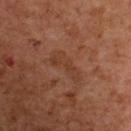workup: total-body-photography surveillance lesion; no biopsy | body site: the upper back | lesion size: ≈4.5 mm | patient: female, aged 58–62 | TBP lesion metrics: roughly 5 lightness units darker than nearby skin and a normalized border contrast of about 5; border irregularity of about 6.5 on a 0–10 scale and peripheral color asymmetry of about 0; a lesion-detection confidence of about 100/100 | tile lighting: cross-polarized | image source: ~15 mm crop, total-body skin-cancer survey.